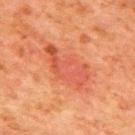  biopsy_status: not biopsied; imaged during a skin examination
  lesion_size:
    long_diameter_mm_approx: 6.5
  site: mid back
  lighting: cross-polarized
  patient:
    sex: male
    age_approx: 80
  image:
    source: total-body photography crop
    field_of_view_mm: 15